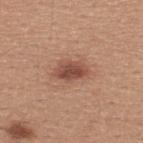biopsy_status: not biopsied; imaged during a skin examination
lesion_size:
  long_diameter_mm_approx: 3.5
patient:
  sex: male
  age_approx: 30
lighting: white-light
image:
  source: total-body photography crop
  field_of_view_mm: 15
automated_metrics:
  eccentricity: 0.8
  shape_asymmetry: 0.25
  border_irregularity_0_10: 2.5
  color_variation_0_10: 3.0
  peripheral_color_asymmetry: 1.0
  nevus_likeness_0_100: 90
  lesion_detection_confidence_0_100: 100
site: upper back